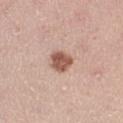| feature | finding |
|---|---|
| tile lighting | white-light illumination |
| subject | female, aged 38–42 |
| automated metrics | an area of roughly 6 mm² and a symmetry-axis asymmetry near 0.2; a mean CIELAB color near L≈56 a*≈21 b*≈27, a lesion–skin lightness drop of about 15, and a normalized border contrast of about 9.5; a border-irregularity rating of about 2/10 and a color-variation rating of about 3/10; an automated nevus-likeness rating near 90 out of 100 |
| site | the leg |
| imaging modality | 15 mm crop, total-body photography |